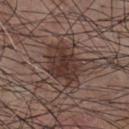Case summary:
- notes — imaged on a skin check; not biopsied
- location — the chest
- imaging modality — ~15 mm tile from a whole-body skin photo
- patient — male, approximately 55 years of age